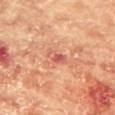{"biopsy_status": "not biopsied; imaged during a skin examination", "image": {"source": "total-body photography crop", "field_of_view_mm": 15}, "lighting": "cross-polarized", "site": "front of the torso", "patient": {"sex": "female", "age_approx": 80}, "lesion_size": {"long_diameter_mm_approx": 3.0}}